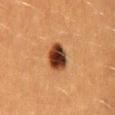<case>
<biopsy_status>not biopsied; imaged during a skin examination</biopsy_status>
<image>
  <source>total-body photography crop</source>
  <field_of_view_mm>15</field_of_view_mm>
</image>
<patient>
  <sex>male</sex>
  <age_approx>40</age_approx>
</patient>
<lesion_size>
  <long_diameter_mm_approx>3.5</long_diameter_mm_approx>
</lesion_size>
<site>back</site>
</case>